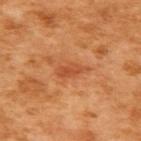biopsy status: total-body-photography surveillance lesion; no biopsy
image source: total-body-photography crop, ~15 mm field of view
lighting: cross-polarized illumination
automated metrics: a footprint of about 5 mm²; a mean CIELAB color near L≈54 a*≈31 b*≈42, about 9 CIELAB-L* units darker than the surrounding skin, and a normalized lesion–skin contrast near 6; a border-irregularity index near 3/10, a color-variation rating of about 2.5/10, and a peripheral color-asymmetry measure near 1; a classifier nevus-likeness of about 0/100 and a lesion-detection confidence of about 100/100
subject: female, approximately 55 years of age
anatomic site: the upper back